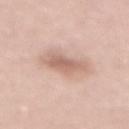<record>
<biopsy_status>not biopsied; imaged during a skin examination</biopsy_status>
<patient>
  <sex>female</sex>
  <age_approx>65</age_approx>
</patient>
<site>chest</site>
<image>
  <source>total-body photography crop</source>
  <field_of_view_mm>15</field_of_view_mm>
</image>
</record>